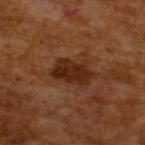biopsy_status: not biopsied; imaged during a skin examination
patient:
  sex: male
  age_approx: 65
lighting: cross-polarized
automated_metrics:
  eccentricity: 0.8
  shape_asymmetry: 0.2
  cielab_L: 26
  cielab_a: 22
  cielab_b: 30
  vs_skin_darker_L: 10.0
  nevus_likeness_0_100: 55
  lesion_detection_confidence_0_100: 100
lesion_size:
  long_diameter_mm_approx: 4.5
image:
  source: total-body photography crop
  field_of_view_mm: 15